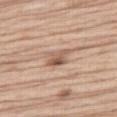Q: Was this lesion biopsied?
A: total-body-photography surveillance lesion; no biopsy
Q: How was this image acquired?
A: ~15 mm crop, total-body skin-cancer survey
Q: Illumination type?
A: white-light illumination
Q: Who is the patient?
A: male, approximately 70 years of age
Q: Automated lesion metrics?
A: a lesion area of about 5 mm²; a border-irregularity rating of about 3/10, a within-lesion color-variation index near 6.5/10, and a peripheral color-asymmetry measure near 2.5; a nevus-likeness score of about 65/100
Q: Where on the body is the lesion?
A: the right thigh